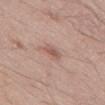{"site": "lower back", "image": {"source": "total-body photography crop", "field_of_view_mm": 15}, "patient": {"sex": "male", "age_approx": 40}, "lesion_size": {"long_diameter_mm_approx": 2.5}, "lighting": "white-light"}